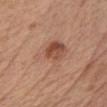Assessment:
Part of a total-body skin-imaging series; this lesion was reviewed on a skin check and was not flagged for biopsy.
Image and clinical context:
The lesion is located on the chest. Imaged with white-light lighting. A 15 mm close-up tile from a total-body photography series done for melanoma screening. The total-body-photography lesion software estimated a lesion area of about 16 mm², an eccentricity of roughly 0.7, and two-axis asymmetry of about 0.3. The analysis additionally found a mean CIELAB color near L≈53 a*≈21 b*≈29, a lesion–skin lightness drop of about 7, and a lesion-to-skin contrast of about 5.5 (normalized; higher = more distinct). The patient is a female aged approximately 65.